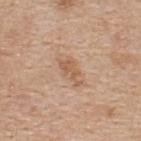{"biopsy_status": "not biopsied; imaged during a skin examination", "site": "upper back", "image": {"source": "total-body photography crop", "field_of_view_mm": 15}, "automated_metrics": {"area_mm2_approx": 4.5, "cielab_L": 58, "cielab_a": 20, "cielab_b": 32, "vs_skin_darker_L": 9.0, "color_variation_0_10": 1.5, "peripheral_color_asymmetry": 0.5, "nevus_likeness_0_100": 5, "lesion_detection_confidence_0_100": 100}, "patient": {"sex": "male", "age_approx": 60}, "lighting": "white-light", "lesion_size": {"long_diameter_mm_approx": 3.5}}